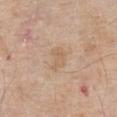Findings:
• workup · catalogued during a skin exam; not biopsied
• acquisition · ~15 mm tile from a whole-body skin photo
• TBP lesion metrics · about 6 CIELAB-L* units darker than the surrounding skin and a normalized border contrast of about 5; an automated nevus-likeness rating near 0 out of 100 and a lesion-detection confidence of about 100/100
• illumination · white-light
• patient · male, aged around 80
• body site · the chest
• size · about 3 mm